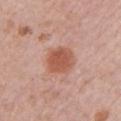Recorded during total-body skin imaging; not selected for excision or biopsy. A female patient roughly 60 years of age. Located on the left upper arm. This image is a 15 mm lesion crop taken from a total-body photograph.